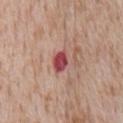Q: Is there a histopathology result?
A: catalogued during a skin exam; not biopsied
Q: What are the patient's age and sex?
A: male, aged approximately 65
Q: Where on the body is the lesion?
A: the chest
Q: What is the imaging modality?
A: total-body-photography crop, ~15 mm field of view
Q: Lesion size?
A: about 3 mm
Q: Automated lesion metrics?
A: a mean CIELAB color near L≈49 a*≈31 b*≈22, about 14 CIELAB-L* units darker than the surrounding skin, and a normalized lesion–skin contrast near 10
Q: What lighting was used for the tile?
A: white-light illumination